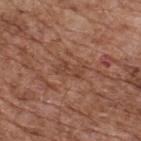Impression: The lesion was photographed on a routine skin check and not biopsied; there is no pathology result. Acquisition and patient details: Measured at roughly 3 mm in maximum diameter. The tile uses white-light illumination. A male patient, aged around 75. On the back. A close-up tile cropped from a whole-body skin photograph, about 15 mm across.